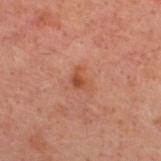Case summary:
– workup · catalogued during a skin exam; not biopsied
– lesion diameter · ~2.5 mm (longest diameter)
– imaging modality · ~15 mm crop, total-body skin-cancer survey
– image-analysis metrics · an average lesion color of about L≈40 a*≈22 b*≈28 (CIELAB), a lesion–skin lightness drop of about 7, and a normalized lesion–skin contrast near 6.5; a border-irregularity index near 3.5/10 and a color-variation rating of about 2.5/10
– anatomic site · the upper back
– subject · male, aged approximately 40